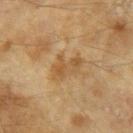Captured during whole-body skin photography for melanoma surveillance; the lesion was not biopsied.
Measured at roughly 4 mm in maximum diameter.
A female patient about 60 years old.
Automated tile analysis of the lesion measured an average lesion color of about L≈49 a*≈16 b*≈36 (CIELAB), about 7 CIELAB-L* units darker than the surrounding skin, and a lesion-to-skin contrast of about 6 (normalized; higher = more distinct). And it measured a within-lesion color-variation index near 4/10 and a peripheral color-asymmetry measure near 1.
A lesion tile, about 15 mm wide, cut from a 3D total-body photograph.
Located on the arm.